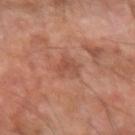Clinical impression: Captured during whole-body skin photography for melanoma surveillance; the lesion was not biopsied. Image and clinical context: A 15 mm close-up tile from a total-body photography series done for melanoma screening. The patient is a male in their 60s. Captured under cross-polarized illumination. From the left forearm. The lesion's longest dimension is about 3 mm.